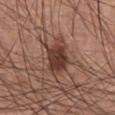| field | value |
|---|---|
| notes | imaged on a skin check; not biopsied |
| patient | male, aged 63–67 |
| diameter | about 4 mm |
| imaging modality | ~15 mm crop, total-body skin-cancer survey |
| location | the front of the torso |
| TBP lesion metrics | a lesion area of about 10 mm², an eccentricity of roughly 0.65, and two-axis asymmetry of about 0.2; a mean CIELAB color near L≈38 a*≈20 b*≈24 and a lesion–skin lightness drop of about 13 |